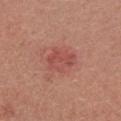Q: Was a biopsy performed?
A: catalogued during a skin exam; not biopsied
Q: What is the anatomic site?
A: the right upper arm
Q: Who is the patient?
A: female, approximately 25 years of age
Q: How was this image acquired?
A: total-body-photography crop, ~15 mm field of view
Q: Illumination type?
A: white-light
Q: Lesion size?
A: ≈4 mm